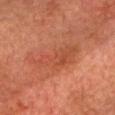biopsy_status: not biopsied; imaged during a skin examination
image:
  source: total-body photography crop
  field_of_view_mm: 15
patient:
  sex: male
  age_approx: 75
site: front of the torso